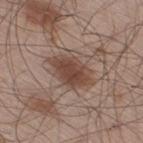Imaged during a routine full-body skin examination; the lesion was not biopsied and no histopathology is available. A roughly 15 mm field-of-view crop from a total-body skin photograph. Measured at roughly 5.5 mm in maximum diameter. The tile uses white-light illumination. From the leg. The patient is a male aged 58 to 62.